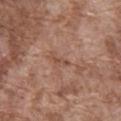This lesion was catalogued during total-body skin photography and was not selected for biopsy.
The tile uses white-light illumination.
Approximately 2.5 mm at its widest.
A close-up tile cropped from a whole-body skin photograph, about 15 mm across.
On the abdomen.
The patient is a male aged around 75.